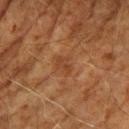No biopsy was performed on this lesion — it was imaged during a full skin examination and was not determined to be concerning.
The lesion-visualizer software estimated a footprint of about 4 mm², an outline eccentricity of about 0.7 (0 = round, 1 = elongated), and two-axis asymmetry of about 0.3. The software also gave a mean CIELAB color near L≈35 a*≈20 b*≈30 and about 5 CIELAB-L* units darker than the surrounding skin. And it measured a classifier nevus-likeness of about 0/100 and a detector confidence of about 100 out of 100 that the crop contains a lesion.
From the left upper arm.
This is a cross-polarized tile.
A 15 mm close-up extracted from a 3D total-body photography capture.
Longest diameter approximately 2.5 mm.
The patient is a male in their 70s.